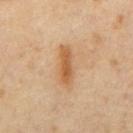biopsy_status: not biopsied; imaged during a skin examination
patient:
  sex: male
  age_approx: 65
lighting: cross-polarized
lesion_size:
  long_diameter_mm_approx: 5.0
image:
  source: total-body photography crop
  field_of_view_mm: 15